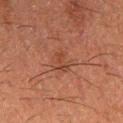A lesion tile, about 15 mm wide, cut from a 3D total-body photograph. A male patient approximately 50 years of age. Measured at roughly 2.5 mm in maximum diameter. Automated tile analysis of the lesion measured a within-lesion color-variation index near 0/10 and a peripheral color-asymmetry measure near 0. Located on the right upper arm. The tile uses cross-polarized illumination.